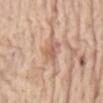This lesion was catalogued during total-body skin photography and was not selected for biopsy. A 15 mm close-up extracted from a 3D total-body photography capture. Automated image analysis of the tile measured a border-irregularity index near 3.5/10 and a within-lesion color-variation index near 2.5/10. And it measured a classifier nevus-likeness of about 0/100 and a lesion-detection confidence of about 100/100. From the abdomen. Captured under white-light illumination. The patient is a female roughly 65 years of age.